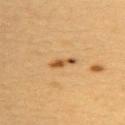Part of a total-body skin-imaging series; this lesion was reviewed on a skin check and was not flagged for biopsy. The recorded lesion diameter is about 3.5 mm. Located on the upper back. Imaged with cross-polarized lighting. Cropped from a whole-body photographic skin survey; the tile spans about 15 mm. The subject is a female approximately 30 years of age. An algorithmic analysis of the crop reported an average lesion color of about L≈51 a*≈19 b*≈39 (CIELAB), roughly 11 lightness units darker than nearby skin, and a normalized border contrast of about 8. The software also gave a border-irregularity rating of about 3.5/10, a within-lesion color-variation index near 2/10, and radial color variation of about 0.